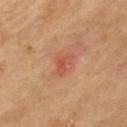Clinical impression:
Recorded during total-body skin imaging; not selected for excision or biopsy.
Context:
The tile uses cross-polarized illumination. Located on the chest. An algorithmic analysis of the crop reported an average lesion color of about L≈41 a*≈23 b*≈27 (CIELAB) and a lesion–skin lightness drop of about 6. It also reported a border-irregularity index near 5/10, internal color variation of about 0 on a 0–10 scale, and radial color variation of about 0. A 15 mm close-up extracted from a 3D total-body photography capture. A male patient, aged 43–47. The recorded lesion diameter is about 3 mm.